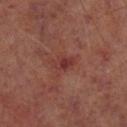workup: total-body-photography surveillance lesion; no biopsy | image: 15 mm crop, total-body photography | anatomic site: the leg | image-analysis metrics: a lesion area of about 4 mm², an eccentricity of roughly 0.8, and a symmetry-axis asymmetry near 0.3; a mean CIELAB color near L≈35 a*≈27 b*≈23, roughly 7 lightness units darker than nearby skin, and a normalized lesion–skin contrast near 6.5 | patient: male, aged 63 to 67 | diameter: ≈2.5 mm.